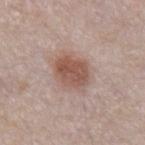The lesion was photographed on a routine skin check and not biopsied; there is no pathology result. The patient is a male in their mid- to late 50s. This image is a 15 mm lesion crop taken from a total-body photograph. Captured under white-light illumination. Located on the abdomen.